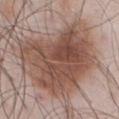Case summary:
– workup: no biopsy performed (imaged during a skin exam)
– image: ~15 mm crop, total-body skin-cancer survey
– subject: male, about 55 years old
– illumination: white-light illumination
– size: ≈9 mm
– site: the front of the torso